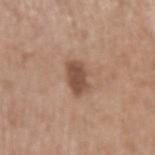Image and clinical context: Captured under white-light illumination. A 15 mm crop from a total-body photograph taken for skin-cancer surveillance. An algorithmic analysis of the crop reported a lesion–skin lightness drop of about 12 and a lesion-to-skin contrast of about 8.5 (normalized; higher = more distinct). A male patient approximately 50 years of age. The lesion's longest dimension is about 3.5 mm. On the left upper arm.